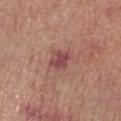workup — catalogued during a skin exam; not biopsied | diameter — about 3 mm | image-analysis metrics — a border-irregularity rating of about 2.5/10, internal color variation of about 2.5 on a 0–10 scale, and radial color variation of about 1 | patient — female, in their mid- to late 60s | acquisition — total-body-photography crop, ~15 mm field of view | illumination — white-light illumination | site — the left forearm.